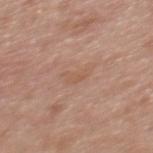notes: imaged on a skin check; not biopsied | automated metrics: a lesion color around L≈56 a*≈19 b*≈30 in CIELAB and a normalized lesion–skin contrast near 4.5; border irregularity of about 5 on a 0–10 scale, internal color variation of about 0.5 on a 0–10 scale, and radial color variation of about 0; a lesion-detection confidence of about 100/100 | illumination: white-light | body site: the upper back | image source: ~15 mm crop, total-body skin-cancer survey | lesion diameter: about 3 mm | subject: female, in their mid- to late 30s.